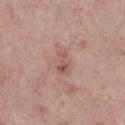<record>
<biopsy_status>not biopsied; imaged during a skin examination</biopsy_status>
<image>
  <source>total-body photography crop</source>
  <field_of_view_mm>15</field_of_view_mm>
</image>
<patient>
  <sex>female</sex>
  <age_approx>65</age_approx>
</patient>
<site>left lower leg</site>
<automated_metrics>
  <area_mm2_approx>4.5</area_mm2_approx>
  <eccentricity>0.75</eccentricity>
  <shape_asymmetry>0.3</shape_asymmetry>
  <vs_skin_darker_L>8.0</vs_skin_darker_L>
</automated_metrics>
</record>